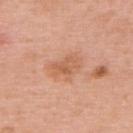<case>
<biopsy_status>not biopsied; imaged during a skin examination</biopsy_status>
<automated_metrics>
  <cielab_L>60</cielab_L>
  <cielab_a>24</cielab_a>
  <cielab_b>35</cielab_b>
  <vs_skin_darker_L>8.0</vs_skin_darker_L>
  <vs_skin_contrast_norm>6.0</vs_skin_contrast_norm>
  <nevus_likeness_0_100>0</nevus_likeness_0_100>
  <lesion_detection_confidence_0_100>100</lesion_detection_confidence_0_100>
</automated_metrics>
<image>
  <source>total-body photography crop</source>
  <field_of_view_mm>15</field_of_view_mm>
</image>
<patient>
  <sex>male</sex>
  <age_approx>80</age_approx>
</patient>
<lighting>white-light</lighting>
<site>upper back</site>
</case>